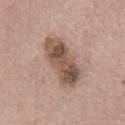biopsy status: catalogued during a skin exam; not biopsied | body site: the abdomen | size: ~7.5 mm (longest diameter) | lighting: white-light illumination | subject: male, aged 73–77 | image source: ~15 mm crop, total-body skin-cancer survey.